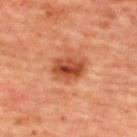Recorded during total-body skin imaging; not selected for excision or biopsy. The lesion is located on the upper back. This is a cross-polarized tile. A roughly 15 mm field-of-view crop from a total-body skin photograph. The patient is a female aged 43 to 47.